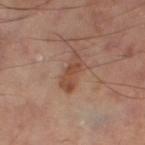Clinical summary:
Longest diameter approximately 5 mm. The lesion-visualizer software estimated a lesion color around L≈48 a*≈21 b*≈29 in CIELAB. The software also gave border irregularity of about 4.5 on a 0–10 scale, a within-lesion color-variation index near 3/10, and radial color variation of about 1. A region of skin cropped from a whole-body photographic capture, roughly 15 mm wide. Imaged with cross-polarized lighting. Located on the right thigh.